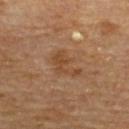The lesion is on the upper back. This is a cross-polarized tile. The patient is a male aged approximately 85 or older. Cropped from a whole-body photographic skin survey; the tile spans about 15 mm.